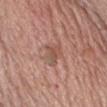<case>
<lesion_size>
  <long_diameter_mm_approx>3.5</long_diameter_mm_approx>
</lesion_size>
<lighting>white-light</lighting>
<image>
  <source>total-body photography crop</source>
  <field_of_view_mm>15</field_of_view_mm>
</image>
<patient>
  <sex>male</sex>
  <age_approx>60</age_approx>
</patient>
<site>chest</site>
</case>